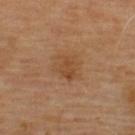Impression:
Recorded during total-body skin imaging; not selected for excision or biopsy.
Background:
The subject is a male aged 68–72. Located on the upper back. A close-up tile cropped from a whole-body skin photograph, about 15 mm across.